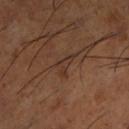No biopsy was performed on this lesion — it was imaged during a full skin examination and was not determined to be concerning. A region of skin cropped from a whole-body photographic capture, roughly 15 mm wide. The total-body-photography lesion software estimated an average lesion color of about L≈33 a*≈17 b*≈25 (CIELAB) and about 6 CIELAB-L* units darker than the surrounding skin. And it measured internal color variation of about 2 on a 0–10 scale and peripheral color asymmetry of about 0.5. The patient is a male aged around 65. The lesion's longest dimension is about 3 mm. The lesion is located on the left lower leg.